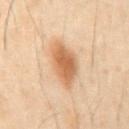follow-up: catalogued during a skin exam; not biopsied
image source: ~15 mm tile from a whole-body skin photo
body site: the front of the torso
patient: male, about 50 years old